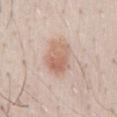– acquisition: 15 mm crop, total-body photography
– automated lesion analysis: an average lesion color of about L≈64 a*≈19 b*≈28 (CIELAB), roughly 10 lightness units darker than nearby skin, and a normalized lesion–skin contrast near 7; a nevus-likeness score of about 95/100
– subject: male, approximately 30 years of age
– anatomic site: the front of the torso
– size: ≈4.5 mm
– illumination: white-light illumination